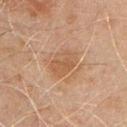Case summary:
– workup: imaged on a skin check; not biopsied